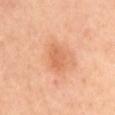Q: Was this lesion biopsied?
A: imaged on a skin check; not biopsied
Q: What did automated image analysis measure?
A: a footprint of about 5.5 mm², an outline eccentricity of about 0.85 (0 = round, 1 = elongated), and a symmetry-axis asymmetry near 0.2
Q: Lesion location?
A: the mid back
Q: What is the lesion's diameter?
A: ~3.5 mm (longest diameter)
Q: What kind of image is this?
A: total-body-photography crop, ~15 mm field of view